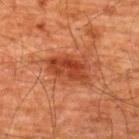{"biopsy_status": "not biopsied; imaged during a skin examination", "site": "upper back", "image": {"source": "total-body photography crop", "field_of_view_mm": 15}, "patient": {"sex": "male", "age_approx": 60}, "lesion_size": {"long_diameter_mm_approx": 5.5}, "automated_metrics": {"area_mm2_approx": 14.0, "eccentricity": 0.8, "nevus_likeness_0_100": 90}, "lighting": "cross-polarized"}